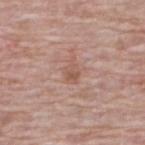• biopsy status: total-body-photography surveillance lesion; no biopsy
• image source: total-body-photography crop, ~15 mm field of view
• body site: the upper back
• patient: male, aged around 65